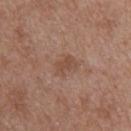notes = imaged on a skin check; not biopsied
patient = female, aged 38 to 42
imaging modality = ~15 mm tile from a whole-body skin photo
anatomic site = the upper back
diameter = about 3 mm